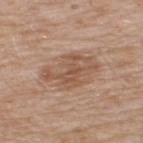Q: Is there a histopathology result?
A: total-body-photography surveillance lesion; no biopsy
Q: What is the anatomic site?
A: the upper back
Q: Illumination type?
A: white-light
Q: Lesion size?
A: about 6 mm
Q: What kind of image is this?
A: ~15 mm tile from a whole-body skin photo
Q: What are the patient's age and sex?
A: male, approximately 65 years of age